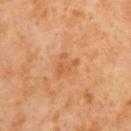Part of a total-body skin-imaging series; this lesion was reviewed on a skin check and was not flagged for biopsy. The total-body-photography lesion software estimated a border-irregularity index near 7.5/10 and a color-variation rating of about 1.5/10. Measured at roughly 3 mm in maximum diameter. This is a cross-polarized tile. The patient is a male in their mid-60s. A 15 mm close-up tile from a total-body photography series done for melanoma screening.